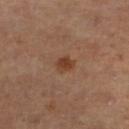Captured during whole-body skin photography for melanoma surveillance; the lesion was not biopsied.
Located on the left lower leg.
A female patient aged around 40.
Captured under cross-polarized illumination.
Approximately 2 mm at its widest.
A region of skin cropped from a whole-body photographic capture, roughly 15 mm wide.